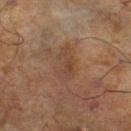{
  "biopsy_status": "not biopsied; imaged during a skin examination",
  "lighting": "cross-polarized",
  "image": {
    "source": "total-body photography crop",
    "field_of_view_mm": 15
  },
  "patient": {
    "sex": "male",
    "age_approx": 70
  },
  "site": "leg"
}